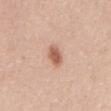Clinical impression: The lesion was photographed on a routine skin check and not biopsied; there is no pathology result. Image and clinical context: A male patient, aged 48–52. This image is a 15 mm lesion crop taken from a total-body photograph. The lesion is on the back.